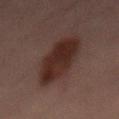Imaged during a routine full-body skin examination; the lesion was not biopsied and no histopathology is available.
Longest diameter approximately 7.5 mm.
The tile uses cross-polarized illumination.
The patient is a male approximately 30 years of age.
On the mid back.
The lesion-visualizer software estimated about 9 CIELAB-L* units darker than the surrounding skin and a lesion-to-skin contrast of about 11 (normalized; higher = more distinct). The software also gave border irregularity of about 2.5 on a 0–10 scale, internal color variation of about 3 on a 0–10 scale, and radial color variation of about 1.
Cropped from a total-body skin-imaging series; the visible field is about 15 mm.